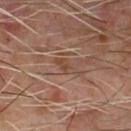Q: Was a biopsy performed?
A: catalogued during a skin exam; not biopsied
Q: What lighting was used for the tile?
A: cross-polarized illumination
Q: Lesion location?
A: the chest
Q: What did automated image analysis measure?
A: a lesion area of about 3 mm² and a shape eccentricity near 0.85; a normalized lesion–skin contrast near 6
Q: What is the imaging modality?
A: ~15 mm tile from a whole-body skin photo
Q: Who is the patient?
A: male, approximately 60 years of age
Q: Lesion size?
A: about 2.5 mm